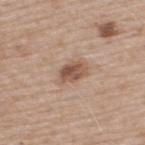* workup: total-body-photography surveillance lesion; no biopsy
* patient: male, aged 63 to 67
* acquisition: ~15 mm tile from a whole-body skin photo
* site: the back